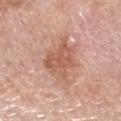image source: 15 mm crop, total-body photography | lesion size: ~5 mm (longest diameter) | lighting: white-light | automated metrics: an eccentricity of roughly 0.65 and two-axis asymmetry of about 0.3; an average lesion color of about L≈58 a*≈23 b*≈31 (CIELAB) and a lesion–skin lightness drop of about 10; lesion-presence confidence of about 100/100 | subject: female, aged approximately 65 | location: the right forearm.